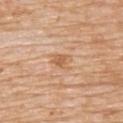Recorded during total-body skin imaging; not selected for excision or biopsy.
A 15 mm close-up tile from a total-body photography series done for melanoma screening.
A male patient, in their mid- to late 70s.
About 2.5 mm across.
Located on the upper back.
The lesion-visualizer software estimated a lesion area of about 4 mm² and a symmetry-axis asymmetry near 0.25. The analysis additionally found border irregularity of about 2.5 on a 0–10 scale.
Imaged with white-light lighting.